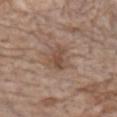  biopsy_status: not biopsied; imaged during a skin examination
  patient:
    sex: male
    age_approx: 85+
  image:
    source: total-body photography crop
    field_of_view_mm: 15
  site: chest
  lighting: white-light
  lesion_size:
    long_diameter_mm_approx: 3.5